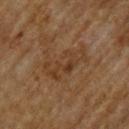Part of a total-body skin-imaging series; this lesion was reviewed on a skin check and was not flagged for biopsy. From the upper back. Imaged with cross-polarized lighting. Cropped from a whole-body photographic skin survey; the tile spans about 15 mm. The patient is a male aged 63 to 67. Approximately 5.5 mm at its widest.